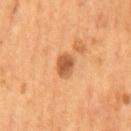Imaged during a routine full-body skin examination; the lesion was not biopsied and no histopathology is available. Cropped from a total-body skin-imaging series; the visible field is about 15 mm. The lesion is located on the mid back. A male subject, in their mid-50s. Captured under cross-polarized illumination.